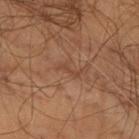Findings:
- image-analysis metrics: border irregularity of about 5 on a 0–10 scale, a within-lesion color-variation index near 0/10, and a peripheral color-asymmetry measure near 0
- lighting: cross-polarized
- diameter: ≈3 mm
- image: ~15 mm tile from a whole-body skin photo
- subject: male, approximately 70 years of age
- body site: the chest